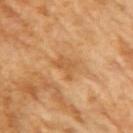A roughly 15 mm field-of-view crop from a total-body skin photograph.
A female patient, approximately 55 years of age.
An algorithmic analysis of the crop reported a lesion area of about 4.5 mm². It also reported a lesion color around L≈59 a*≈23 b*≈43 in CIELAB, a lesion–skin lightness drop of about 7, and a normalized border contrast of about 5. The software also gave a color-variation rating of about 2/10 and peripheral color asymmetry of about 0.5. The analysis additionally found an automated nevus-likeness rating near 0 out of 100 and a detector confidence of about 100 out of 100 that the crop contains a lesion.
Measured at roughly 3 mm in maximum diameter.
This is a cross-polarized tile.
On the right upper arm.